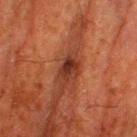Findings:
• follow-up: no biopsy performed (imaged during a skin exam)
• subject: male, about 80 years old
• size: ~2.5 mm (longest diameter)
• imaging modality: ~15 mm crop, total-body skin-cancer survey
• body site: the right thigh
• lighting: cross-polarized illumination